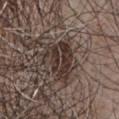Captured during whole-body skin photography for melanoma surveillance; the lesion was not biopsied. An algorithmic analysis of the crop reported a symmetry-axis asymmetry near 0.35. It also reported an automated nevus-likeness rating near 50 out of 100. A lesion tile, about 15 mm wide, cut from a 3D total-body photograph. The tile uses white-light illumination. A male subject, aged approximately 65. On the chest. About 5 mm across.